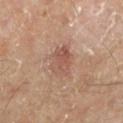Imaged during a routine full-body skin examination; the lesion was not biopsied and no histopathology is available. The tile uses cross-polarized illumination. The total-body-photography lesion software estimated a lesion color around L≈52 a*≈21 b*≈27 in CIELAB, a lesion–skin lightness drop of about 8, and a lesion-to-skin contrast of about 6 (normalized; higher = more distinct). The lesion is located on the leg. The subject is in their mid- to late 50s. Longest diameter approximately 3.5 mm. A region of skin cropped from a whole-body photographic capture, roughly 15 mm wide.